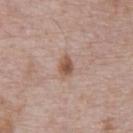Assessment:
Recorded during total-body skin imaging; not selected for excision or biopsy.
Image and clinical context:
The lesion's longest dimension is about 3 mm. A 15 mm close-up extracted from a 3D total-body photography capture. The patient is a male roughly 70 years of age. From the front of the torso. Automated image analysis of the tile measured an average lesion color of about L≈54 a*≈19 b*≈28 (CIELAB), a lesion–skin lightness drop of about 11, and a lesion-to-skin contrast of about 8 (normalized; higher = more distinct).